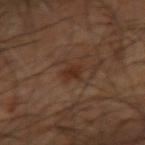Case summary:
* workup — total-body-photography surveillance lesion; no biopsy
* imaging modality — ~15 mm tile from a whole-body skin photo
* illumination — cross-polarized
* anatomic site — the right upper arm
* subject — aged around 65